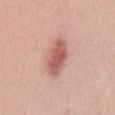{"biopsy_status": "not biopsied; imaged during a skin examination", "lighting": "white-light", "image": {"source": "total-body photography crop", "field_of_view_mm": 15}, "site": "abdomen", "patient": {"sex": "male", "age_approx": 55}}